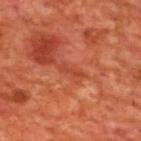biopsy status: catalogued during a skin exam; not biopsied | lesion size: ~3 mm (longest diameter) | site: the upper back | image: 15 mm crop, total-body photography | tile lighting: cross-polarized illumination | automated lesion analysis: a footprint of about 2 mm²; a lesion color around L≈44 a*≈34 b*≈37 in CIELAB, roughly 7 lightness units darker than nearby skin, and a normalized lesion–skin contrast near 5.5; a border-irregularity index near 6/10; an automated nevus-likeness rating near 0 out of 100 and lesion-presence confidence of about 80/100 | subject: male, aged 68–72.